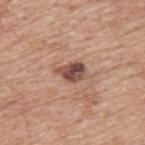This lesion was catalogued during total-body skin photography and was not selected for biopsy. A 15 mm crop from a total-body photograph taken for skin-cancer surveillance. The lesion is located on the upper back. Measured at roughly 3 mm in maximum diameter. The subject is a male aged around 75. Automated image analysis of the tile measured a lesion color around L≈48 a*≈21 b*≈25 in CIELAB, about 15 CIELAB-L* units darker than the surrounding skin, and a lesion-to-skin contrast of about 11 (normalized; higher = more distinct). The analysis additionally found border irregularity of about 3 on a 0–10 scale, a within-lesion color-variation index near 6.5/10, and peripheral color asymmetry of about 2.5. The software also gave a nevus-likeness score of about 75/100 and a lesion-detection confidence of about 100/100. Imaged with white-light lighting.